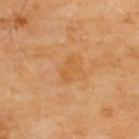- notes — imaged on a skin check; not biopsied
- lesion size — ~3 mm (longest diameter)
- anatomic site — the upper back
- patient — male, aged 68 to 72
- lighting — cross-polarized
- image — total-body-photography crop, ~15 mm field of view